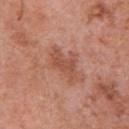<record>
<biopsy_status>not biopsied; imaged during a skin examination</biopsy_status>
<lesion_size>
  <long_diameter_mm_approx>4.0</long_diameter_mm_approx>
</lesion_size>
<site>front of the torso</site>
<patient>
  <sex>male</sex>
  <age_approx>70</age_approx>
</patient>
<image>
  <source>total-body photography crop</source>
  <field_of_view_mm>15</field_of_view_mm>
</image>
</record>